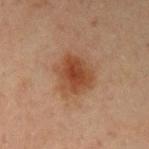Recorded during total-body skin imaging; not selected for excision or biopsy.
The lesion is located on the left upper arm.
A close-up tile cropped from a whole-body skin photograph, about 15 mm across.
A male patient, roughly 45 years of age.